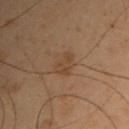No biopsy was performed on this lesion — it was imaged during a full skin examination and was not determined to be concerning. A male patient, aged around 55. A 15 mm close-up tile from a total-body photography series done for melanoma screening. Approximately 2.5 mm at its widest. The lesion is on the left upper arm. An algorithmic analysis of the crop reported an area of roughly 4 mm², an eccentricity of roughly 0.7, and a shape-asymmetry score of about 0.5 (0 = symmetric). The software also gave a nevus-likeness score of about 0/100.